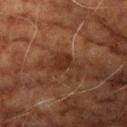workup = catalogued during a skin exam; not biopsied